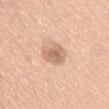follow-up = imaged on a skin check; not biopsied